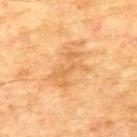Q: Is there a histopathology result?
A: total-body-photography surveillance lesion; no biopsy
Q: Automated lesion metrics?
A: a lesion color around L≈55 a*≈20 b*≈38 in CIELAB and a lesion–skin lightness drop of about 7
Q: What is the anatomic site?
A: the upper back
Q: What is the imaging modality?
A: ~15 mm tile from a whole-body skin photo
Q: How large is the lesion?
A: ~4.5 mm (longest diameter)
Q: Who is the patient?
A: male, aged around 75
Q: How was the tile lit?
A: cross-polarized